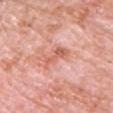<lesion>
<biopsy_status>not biopsied; imaged during a skin examination</biopsy_status>
<site>chest</site>
<automated_metrics>
  <area_mm2_approx>5.0</area_mm2_approx>
  <shape_asymmetry>0.45</shape_asymmetry>
  <cielab_L>62</cielab_L>
  <cielab_a>28</cielab_a>
  <cielab_b>31</cielab_b>
  <vs_skin_darker_L>9.0</vs_skin_darker_L>
  <vs_skin_contrast_norm>6.5</vs_skin_contrast_norm>
  <nevus_likeness_0_100>0</nevus_likeness_0_100>
  <lesion_detection_confidence_0_100>100</lesion_detection_confidence_0_100>
</automated_metrics>
<image>
  <source>total-body photography crop</source>
  <field_of_view_mm>15</field_of_view_mm>
</image>
<patient>
  <sex>male</sex>
  <age_approx>80</age_approx>
</patient>
<lesion_size>
  <long_diameter_mm_approx>3.5</long_diameter_mm_approx>
</lesion_size>
<lighting>white-light</lighting>
</lesion>